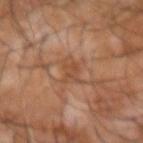| field | value |
|---|---|
| follow-up | total-body-photography surveillance lesion; no biopsy |
| lesion diameter | about 3 mm |
| site | the right forearm |
| illumination | cross-polarized illumination |
| patient | male, aged approximately 55 |
| automated metrics | a within-lesion color-variation index near 2/10 and a peripheral color-asymmetry measure near 0.5 |
| image source | ~15 mm crop, total-body skin-cancer survey |